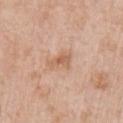Recorded during total-body skin imaging; not selected for excision or biopsy.
A male patient aged around 80.
Measured at roughly 3 mm in maximum diameter.
A close-up tile cropped from a whole-body skin photograph, about 15 mm across.
On the front of the torso.
The tile uses white-light illumination.
An algorithmic analysis of the crop reported a lesion area of about 5 mm² and an outline eccentricity of about 0.8 (0 = round, 1 = elongated). The analysis additionally found a border-irregularity index near 3.5/10, a color-variation rating of about 2.5/10, and a peripheral color-asymmetry measure near 0.5. The analysis additionally found an automated nevus-likeness rating near 0 out of 100 and lesion-presence confidence of about 100/100.